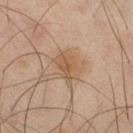A 15 mm crop from a total-body photograph taken for skin-cancer surveillance. A male subject, aged 43 to 47. Automated image analysis of the tile measured an average lesion color of about L≈43 a*≈14 b*≈27 (CIELAB) and a normalized border contrast of about 6.5. Captured under cross-polarized illumination. On the right thigh. About 4 mm across.